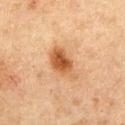Clinical summary: On the abdomen. The lesion-visualizer software estimated an area of roughly 8.5 mm², an outline eccentricity of about 0.8 (0 = round, 1 = elongated), and two-axis asymmetry of about 0.25. The analysis additionally found a mean CIELAB color near L≈45 a*≈21 b*≈34 and a lesion-to-skin contrast of about 9.5 (normalized; higher = more distinct). A 15 mm close-up extracted from a 3D total-body photography capture. A male patient, aged 73–77. The tile uses cross-polarized illumination. Measured at roughly 4.5 mm in maximum diameter.